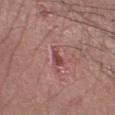Captured during whole-body skin photography for melanoma surveillance; the lesion was not biopsied. The tile uses white-light illumination. The lesion's longest dimension is about 2.5 mm. Automated image analysis of the tile measured an area of roughly 3 mm², a shape eccentricity near 0.85, and a symmetry-axis asymmetry near 0.4. The analysis additionally found a nevus-likeness score of about 0/100 and lesion-presence confidence of about 100/100. A 15 mm close-up extracted from a 3D total-body photography capture. The patient is a male in their mid- to late 40s. On the right forearm.